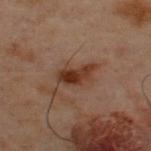{
  "biopsy_status": "not biopsied; imaged during a skin examination",
  "patient": {
    "sex": "male",
    "age_approx": 50
  },
  "lesion_size": {
    "long_diameter_mm_approx": 4.0
  },
  "image": {
    "source": "total-body photography crop",
    "field_of_view_mm": 15
  },
  "site": "upper back"
}